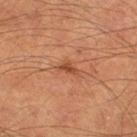Case summary:
• notes · imaged on a skin check; not biopsied
• subject · male, approximately 65 years of age
• diameter · ~3 mm (longest diameter)
• site · the right thigh
• image · ~15 mm tile from a whole-body skin photo
• tile lighting · cross-polarized